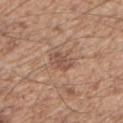Recorded during total-body skin imaging; not selected for excision or biopsy. The lesion is on the arm. This is a white-light tile. A male subject, about 60 years old. Cropped from a total-body skin-imaging series; the visible field is about 15 mm. Automated image analysis of the tile measured a footprint of about 5 mm² and two-axis asymmetry of about 0.2. The software also gave roughly 9 lightness units darker than nearby skin and a normalized lesion–skin contrast near 6.5.